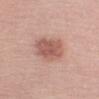The lesion-visualizer software estimated border irregularity of about 2.5 on a 0–10 scale and radial color variation of about 1. The software also gave a classifier nevus-likeness of about 80/100 and lesion-presence confidence of about 100/100. The lesion's longest dimension is about 5 mm. On the right upper arm. A 15 mm close-up tile from a total-body photography series done for melanoma screening. Imaged with white-light lighting. The subject is a male aged 48–52.